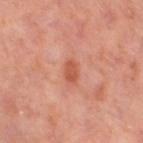<tbp_lesion>
  <biopsy_status>not biopsied; imaged during a skin examination</biopsy_status>
  <automated_metrics>
    <area_mm2_approx>4.0</area_mm2_approx>
    <eccentricity>0.85</eccentricity>
    <shape_asymmetry>0.3</shape_asymmetry>
    <cielab_L>53</cielab_L>
    <cielab_a>29</cielab_a>
    <cielab_b>32</cielab_b>
    <vs_skin_contrast_norm>7.0</vs_skin_contrast_norm>
    <border_irregularity_0_10>2.5</border_irregularity_0_10>
    <color_variation_0_10>1.5</color_variation_0_10>
    <peripheral_color_asymmetry>0.5</peripheral_color_asymmetry>
  </automated_metrics>
  <patient>
    <sex>female</sex>
    <age_approx>50</age_approx>
  </patient>
  <image>
    <source>total-body photography crop</source>
    <field_of_view_mm>15</field_of_view_mm>
  </image>
  <lesion_size>
    <long_diameter_mm_approx>3.0</long_diameter_mm_approx>
  </lesion_size>
  <site>right thigh</site>
</tbp_lesion>